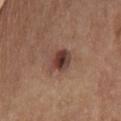Assessment: Part of a total-body skin-imaging series; this lesion was reviewed on a skin check and was not flagged for biopsy. Image and clinical context: Located on the front of the torso. A roughly 15 mm field-of-view crop from a total-body skin photograph. The subject is a female in their mid- to late 60s. This is a white-light tile.